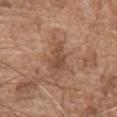Recorded during total-body skin imaging; not selected for excision or biopsy. A male subject in their mid- to late 70s. This is a white-light tile. The lesion is located on the chest. A region of skin cropped from a whole-body photographic capture, roughly 15 mm wide. Longest diameter approximately 4 mm. Automated image analysis of the tile measured a lesion color around L≈48 a*≈20 b*≈30 in CIELAB, roughly 9 lightness units darker than nearby skin, and a normalized lesion–skin contrast near 6.5. The analysis additionally found a border-irregularity index near 5/10. The software also gave a classifier nevus-likeness of about 0/100 and a detector confidence of about 95 out of 100 that the crop contains a lesion.